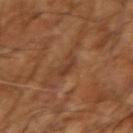Case summary:
– biopsy status — no biopsy performed (imaged during a skin exam)
– imaging modality — total-body-photography crop, ~15 mm field of view
– tile lighting — cross-polarized illumination
– image-analysis metrics — an automated nevus-likeness rating near 0 out of 100
– patient — male, about 55 years old
– lesion diameter — about 2.5 mm
– location — the right upper arm